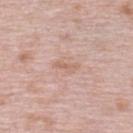{
  "biopsy_status": "not biopsied; imaged during a skin examination",
  "site": "upper back",
  "patient": {
    "sex": "female",
    "age_approx": 65
  },
  "lesion_size": {
    "long_diameter_mm_approx": 2.5
  },
  "lighting": "white-light",
  "image": {
    "source": "total-body photography crop",
    "field_of_view_mm": 15
  }
}